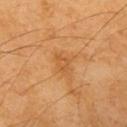{
  "biopsy_status": "not biopsied; imaged during a skin examination",
  "site": "left upper arm",
  "patient": {
    "sex": "male",
    "age_approx": 70
  },
  "lesion_size": {
    "long_diameter_mm_approx": 2.5
  },
  "lighting": "cross-polarized",
  "image": {
    "source": "total-body photography crop",
    "field_of_view_mm": 15
  },
  "automated_metrics": {
    "area_mm2_approx": 3.5,
    "eccentricity": 0.75,
    "shape_asymmetry": 0.5,
    "cielab_L": 52,
    "cielab_a": 23,
    "cielab_b": 42,
    "vs_skin_darker_L": 6.0,
    "vs_skin_contrast_norm": 5.0,
    "color_variation_0_10": 1.5
  }
}